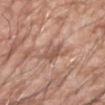Notes:
* follow-up — imaged on a skin check; not biopsied
* patient — male, roughly 60 years of age
* automated metrics — a border-irregularity rating of about 5/10 and peripheral color asymmetry of about 1
* lighting — white-light illumination
* site — the left forearm
* imaging modality — total-body-photography crop, ~15 mm field of view
* size — ≈3.5 mm